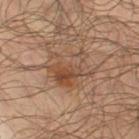{"biopsy_status": "not biopsied; imaged during a skin examination", "patient": {"sex": "male", "age_approx": 65}, "lighting": "cross-polarized", "site": "left thigh", "image": {"source": "total-body photography crop", "field_of_view_mm": 15}, "lesion_size": {"long_diameter_mm_approx": 5.5}}